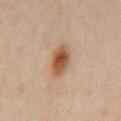The lesion was tiled from a total-body skin photograph and was not biopsied. On the chest. A 15 mm close-up extracted from a 3D total-body photography capture. Automated image analysis of the tile measured an area of roughly 8 mm². The analysis additionally found an average lesion color of about L≈54 a*≈20 b*≈34 (CIELAB). It also reported a color-variation rating of about 5/10 and peripheral color asymmetry of about 1. It also reported an automated nevus-likeness rating near 100 out of 100 and a lesion-detection confidence of about 100/100. A male subject, aged around 65. About 4 mm across.